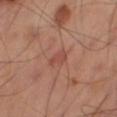Q: Was this lesion biopsied?
A: imaged on a skin check; not biopsied
Q: How was this image acquired?
A: ~15 mm crop, total-body skin-cancer survey
Q: What is the lesion's diameter?
A: about 2.5 mm
Q: Illumination type?
A: cross-polarized illumination
Q: Where on the body is the lesion?
A: the right thigh